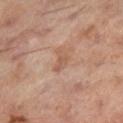Recorded during total-body skin imaging; not selected for excision or biopsy.